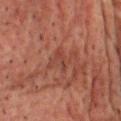No biopsy was performed on this lesion — it was imaged during a full skin examination and was not determined to be concerning. The lesion-visualizer software estimated a lesion area of about 3 mm² and two-axis asymmetry of about 0.45. The software also gave a classifier nevus-likeness of about 0/100. The subject is a male roughly 60 years of age. The lesion is located on the front of the torso. A 15 mm crop from a total-body photograph taken for skin-cancer surveillance.